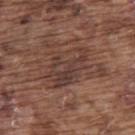Impression: Imaged during a routine full-body skin examination; the lesion was not biopsied and no histopathology is available. Clinical summary: A male subject in their mid-70s. From the upper back. A 15 mm close-up extracted from a 3D total-body photography capture.